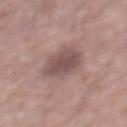  biopsy_status: not biopsied; imaged during a skin examination
  site: mid back
  image:
    source: total-body photography crop
    field_of_view_mm: 15
  patient:
    sex: male
    age_approx: 55